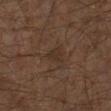Impression: This lesion was catalogued during total-body skin photography and was not selected for biopsy. Context: From the leg. Automated tile analysis of the lesion measured a border-irregularity index near 2.5/10 and a within-lesion color-variation index near 1.5/10. The tile uses cross-polarized illumination. The recorded lesion diameter is about 2.5 mm. A region of skin cropped from a whole-body photographic capture, roughly 15 mm wide. The patient is a male about 60 years old.